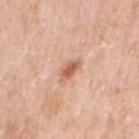The lesion was photographed on a routine skin check and not biopsied; there is no pathology result.
Located on the right upper arm.
A female subject, in their mid-50s.
Cropped from a total-body skin-imaging series; the visible field is about 15 mm.